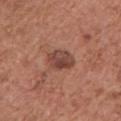Assessment:
This lesion was catalogued during total-body skin photography and was not selected for biopsy.
Image and clinical context:
A region of skin cropped from a whole-body photographic capture, roughly 15 mm wide. On the front of the torso. A male subject about 75 years old.